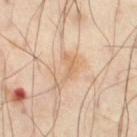  biopsy_status: not biopsied; imaged during a skin examination
  lesion_size:
    long_diameter_mm_approx: 4.5
  lighting: cross-polarized
  patient:
    sex: male
    age_approx: 55
  site: right thigh
  image:
    source: total-body photography crop
    field_of_view_mm: 15